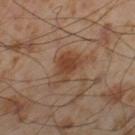Impression:
The lesion was photographed on a routine skin check and not biopsied; there is no pathology result.
Context:
The subject is a male approximately 55 years of age. This is a cross-polarized tile. Measured at roughly 4.5 mm in maximum diameter. Automated image analysis of the tile measured a mean CIELAB color near L≈43 a*≈20 b*≈30, about 8 CIELAB-L* units darker than the surrounding skin, and a normalized lesion–skin contrast near 7.5. The software also gave a border-irregularity index near 4.5/10, a color-variation rating of about 3/10, and radial color variation of about 1. The software also gave an automated nevus-likeness rating near 90 out of 100 and a detector confidence of about 100 out of 100 that the crop contains a lesion. This image is a 15 mm lesion crop taken from a total-body photograph. Located on the left thigh.